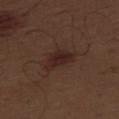Findings:
• anatomic site — the right thigh
• acquisition — total-body-photography crop, ~15 mm field of view
• patient — male, aged approximately 70